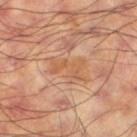Cropped from a whole-body photographic skin survey; the tile spans about 15 mm.
The patient is a male aged approximately 60.
Imaged with cross-polarized lighting.
From the leg.
The lesion's longest dimension is about 4 mm.